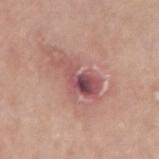Q: Was a biopsy performed?
A: no biopsy performed (imaged during a skin exam)
Q: What kind of image is this?
A: ~15 mm crop, total-body skin-cancer survey
Q: What is the lesion's diameter?
A: ~5 mm (longest diameter)
Q: Who is the patient?
A: male, approximately 55 years of age
Q: Lesion location?
A: the right upper arm
Q: What lighting was used for the tile?
A: white-light
Q: Automated lesion metrics?
A: a footprint of about 10 mm², a shape eccentricity near 0.85, and two-axis asymmetry of about 0.4; about 12 CIELAB-L* units darker than the surrounding skin and a lesion-to-skin contrast of about 8.5 (normalized; higher = more distinct); a border-irregularity rating of about 6/10, internal color variation of about 10 on a 0–10 scale, and radial color variation of about 4; a classifier nevus-likeness of about 0/100 and a lesion-detection confidence of about 100/100